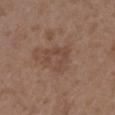  biopsy_status: not biopsied; imaged during a skin examination
  site: arm
  image:
    source: total-body photography crop
    field_of_view_mm: 15
  patient:
    sex: female
    age_approx: 35